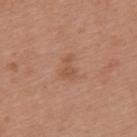Findings:
– notes — imaged on a skin check; not biopsied
– location — the upper back
– lighting — white-light illumination
– lesion size — about 3 mm
– subject — female, roughly 40 years of age
– image — ~15 mm tile from a whole-body skin photo